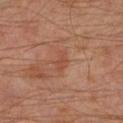Clinical impression: The lesion was photographed on a routine skin check and not biopsied; there is no pathology result. Acquisition and patient details: A 15 mm close-up extracted from a 3D total-body photography capture. On the left lower leg. A male patient, in their 60s.